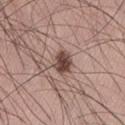Impression:
Imaged during a routine full-body skin examination; the lesion was not biopsied and no histopathology is available.
Acquisition and patient details:
The patient is a male aged around 35. Automated image analysis of the tile measured a footprint of about 5.5 mm². The software also gave a border-irregularity index near 2/10, a within-lesion color-variation index near 4/10, and a peripheral color-asymmetry measure near 1.5. And it measured a nevus-likeness score of about 95/100 and a lesion-detection confidence of about 100/100. The tile uses white-light illumination. This image is a 15 mm lesion crop taken from a total-body photograph. The lesion is on the left thigh.